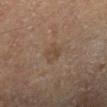Case summary:
– notes — no biopsy performed (imaged during a skin exam)
– location — the left lower leg
– imaging modality — ~15 mm crop, total-body skin-cancer survey
– subject — male, aged around 65